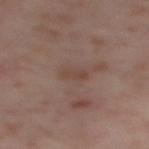The tile uses cross-polarized illumination. A 15 mm crop from a total-body photograph taken for skin-cancer surveillance. An algorithmic analysis of the crop reported an eccentricity of roughly 0.9 and a shape-asymmetry score of about 0.2 (0 = symmetric). And it measured a border-irregularity index near 2.5/10, internal color variation of about 1 on a 0–10 scale, and peripheral color asymmetry of about 0. A female patient in their mid- to late 50s. The lesion is on the leg. Approximately 2.5 mm at its widest.